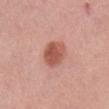Recorded during total-body skin imaging; not selected for excision or biopsy.
On the mid back.
A male subject, about 55 years old.
Automated tile analysis of the lesion measured an area of roughly 9.5 mm² and two-axis asymmetry of about 0.15. The software also gave an average lesion color of about L≈56 a*≈27 b*≈29 (CIELAB), a lesion–skin lightness drop of about 12, and a lesion-to-skin contrast of about 8.5 (normalized; higher = more distinct). The analysis additionally found border irregularity of about 1.5 on a 0–10 scale and radial color variation of about 1.5.
Captured under white-light illumination.
This image is a 15 mm lesion crop taken from a total-body photograph.
Approximately 3.5 mm at its widest.